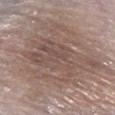Assessment: The lesion was photographed on a routine skin check and not biopsied; there is no pathology result. Image and clinical context: A male subject aged 63 to 67. A close-up tile cropped from a whole-body skin photograph, about 15 mm across. On the right lower leg.